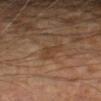Captured during whole-body skin photography for melanoma surveillance; the lesion was not biopsied. The recorded lesion diameter is about 3 mm. This image is a 15 mm lesion crop taken from a total-body photograph. A male subject, aged approximately 70. From the left forearm.